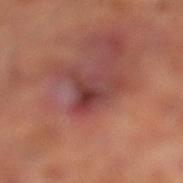No biopsy was performed on this lesion — it was imaged during a full skin examination and was not determined to be concerning.
A male patient, aged 63 to 67.
Cropped from a whole-body photographic skin survey; the tile spans about 15 mm.
Located on the leg.